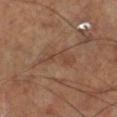Assessment:
Recorded during total-body skin imaging; not selected for excision or biopsy.
Clinical summary:
A male subject aged 68–72. The tile uses cross-polarized illumination. From the leg. A 15 mm close-up extracted from a 3D total-body photography capture. Measured at roughly 5 mm in maximum diameter.